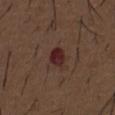{
  "biopsy_status": "not biopsied; imaged during a skin examination",
  "automated_metrics": {
    "area_mm2_approx": 4.5,
    "eccentricity": 0.7,
    "shape_asymmetry": 0.2,
    "border_irregularity_0_10": 1.5,
    "color_variation_0_10": 4.0,
    "nevus_likeness_0_100": 0,
    "lesion_detection_confidence_0_100": 100
  },
  "site": "abdomen",
  "image": {
    "source": "total-body photography crop",
    "field_of_view_mm": 15
  },
  "lighting": "white-light",
  "patient": {
    "sex": "male",
    "age_approx": 50
  },
  "lesion_size": {
    "long_diameter_mm_approx": 3.0
  }
}